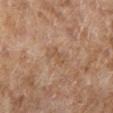Q: Is there a histopathology result?
A: total-body-photography surveillance lesion; no biopsy
Q: Patient demographics?
A: female, aged around 60
Q: Automated lesion metrics?
A: a footprint of about 3.5 mm², a shape eccentricity near 0.8, and a shape-asymmetry score of about 0.4 (0 = symmetric); a border-irregularity index near 5/10, a color-variation rating of about 1/10, and a peripheral color-asymmetry measure near 0
Q: What is the anatomic site?
A: the right lower leg
Q: What lighting was used for the tile?
A: cross-polarized
Q: What kind of image is this?
A: ~15 mm tile from a whole-body skin photo